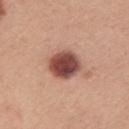Captured during whole-body skin photography for melanoma surveillance; the lesion was not biopsied. Imaged with white-light lighting. Longest diameter approximately 4 mm. The subject is a female aged 43–47. From the arm. Automated tile analysis of the lesion measured an area of roughly 11 mm², an eccentricity of roughly 0.5, and a symmetry-axis asymmetry near 0.1. It also reported a mean CIELAB color near L≈48 a*≈24 b*≈26, roughly 19 lightness units darker than nearby skin, and a normalized lesion–skin contrast near 12.5. It also reported a border-irregularity rating of about 1/10, a color-variation rating of about 6/10, and radial color variation of about 1.5. The software also gave a classifier nevus-likeness of about 90/100 and a lesion-detection confidence of about 100/100. A close-up tile cropped from a whole-body skin photograph, about 15 mm across.